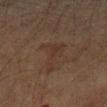Findings:
* follow-up: no biopsy performed (imaged during a skin exam)
* acquisition: 15 mm crop, total-body photography
* body site: the left forearm
* illumination: cross-polarized
* patient: female, approximately 70 years of age
* size: about 4.5 mm